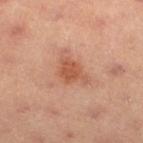Captured during whole-body skin photography for melanoma surveillance; the lesion was not biopsied.
A lesion tile, about 15 mm wide, cut from a 3D total-body photograph.
On the left lower leg.
Captured under cross-polarized illumination.
A male patient aged 53–57.
The recorded lesion diameter is about 3.5 mm.